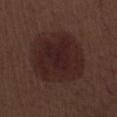<lesion>
<biopsy_status>not biopsied; imaged during a skin examination</biopsy_status>
<lesion_size>
  <long_diameter_mm_approx>6.5</long_diameter_mm_approx>
</lesion_size>
<lighting>white-light</lighting>
<site>right lower leg</site>
<patient>
  <sex>male</sex>
  <age_approx>70</age_approx>
</patient>
<image>
  <source>total-body photography crop</source>
  <field_of_view_mm>15</field_of_view_mm>
</image>
<automated_metrics>
  <area_mm2_approx>35.0</area_mm2_approx>
  <eccentricity>0.4</eccentricity>
  <shape_asymmetry>0.1</shape_asymmetry>
  <cielab_L>22</cielab_L>
  <cielab_a>18</cielab_a>
  <cielab_b>17</cielab_b>
  <vs_skin_darker_L>7.0</vs_skin_darker_L>
  <vs_skin_contrast_norm>9.0</vs_skin_contrast_norm>
  <nevus_likeness_0_100>25</nevus_likeness_0_100>
  <lesion_detection_confidence_0_100>100</lesion_detection_confidence_0_100>
</automated_metrics>
</lesion>